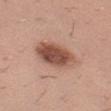Located on the left thigh.
A 15 mm close-up tile from a total-body photography series done for melanoma screening.
Captured under white-light illumination.
Approximately 6 mm at its widest.
A male patient, aged approximately 40.